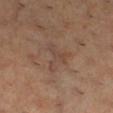Part of a total-body skin-imaging series; this lesion was reviewed on a skin check and was not flagged for biopsy. A female subject aged approximately 30. A roughly 15 mm field-of-view crop from a total-body skin photograph. From the leg. Captured under cross-polarized illumination.